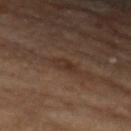Case summary:
- follow-up · imaged on a skin check; not biopsied
- subject · female, in their mid- to late 60s
- acquisition · 15 mm crop, total-body photography
- anatomic site · the left forearm